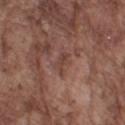Notes:
– follow-up · total-body-photography surveillance lesion; no biopsy
– lighting · white-light illumination
– anatomic site · the left upper arm
– image · ~15 mm crop, total-body skin-cancer survey
– automated metrics · a lesion color around L≈41 a*≈20 b*≈23 in CIELAB and a lesion-to-skin contrast of about 6 (normalized; higher = more distinct); a classifier nevus-likeness of about 0/100 and lesion-presence confidence of about 95/100
– patient · male, roughly 75 years of age
– diameter · ~2.5 mm (longest diameter)